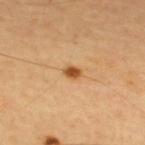Q: Was this lesion biopsied?
A: catalogued during a skin exam; not biopsied
Q: What is the imaging modality?
A: ~15 mm tile from a whole-body skin photo
Q: What lighting was used for the tile?
A: cross-polarized
Q: Who is the patient?
A: male, aged 63–67
Q: Automated lesion metrics?
A: about 14 CIELAB-L* units darker than the surrounding skin and a lesion-to-skin contrast of about 10 (normalized; higher = more distinct); border irregularity of about 1.5 on a 0–10 scale, a within-lesion color-variation index near 2.5/10, and peripheral color asymmetry of about 0.5; a nevus-likeness score of about 100/100 and lesion-presence confidence of about 100/100
Q: What is the anatomic site?
A: the upper back